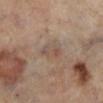- notes: no biopsy performed (imaged during a skin exam)